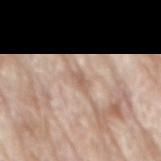Assessment:
This lesion was catalogued during total-body skin photography and was not selected for biopsy.
Clinical summary:
The lesion-visualizer software estimated a border-irregularity index near 3/10, a within-lesion color-variation index near 2/10, and a peripheral color-asymmetry measure near 0.5. From the mid back. A 15 mm close-up tile from a total-body photography series done for melanoma screening. A male subject, aged approximately 80. This is a white-light tile. The recorded lesion diameter is about 2.5 mm.